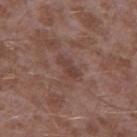Q: Is there a histopathology result?
A: imaged on a skin check; not biopsied
Q: Automated lesion metrics?
A: a lesion color around L≈41 a*≈19 b*≈23 in CIELAB, a lesion–skin lightness drop of about 6, and a normalized border contrast of about 5.5; a border-irregularity index near 4.5/10, a within-lesion color-variation index near 1.5/10, and radial color variation of about 0.5; a nevus-likeness score of about 0/100
Q: Patient demographics?
A: male, approximately 45 years of age
Q: What is the lesion's diameter?
A: about 3.5 mm
Q: Lesion location?
A: the left thigh
Q: How was this image acquired?
A: ~15 mm crop, total-body skin-cancer survey
Q: How was the tile lit?
A: white-light